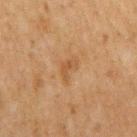workup = no biopsy performed (imaged during a skin exam) | anatomic site = the right upper arm | image source = ~15 mm tile from a whole-body skin photo | patient = male, aged around 50.